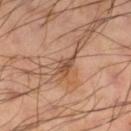{
  "biopsy_status": "not biopsied; imaged during a skin examination",
  "site": "right lower leg",
  "image": {
    "source": "total-body photography crop",
    "field_of_view_mm": 15
  },
  "patient": {
    "sex": "male",
    "age_approx": 50
  }
}